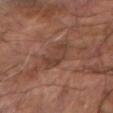Imaged during a routine full-body skin examination; the lesion was not biopsied and no histopathology is available. Located on the right forearm. A male subject aged around 65. A lesion tile, about 15 mm wide, cut from a 3D total-body photograph.